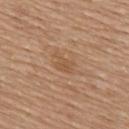Assessment:
This lesion was catalogued during total-body skin photography and was not selected for biopsy.
Background:
A female patient roughly 55 years of age. A 15 mm close-up extracted from a 3D total-body photography capture. The lesion is located on the upper back.